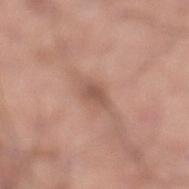Impression: Imaged during a routine full-body skin examination; the lesion was not biopsied and no histopathology is available. Background: The recorded lesion diameter is about 3 mm. Captured under white-light illumination. The lesion is located on the left lower leg. The subject is a male roughly 20 years of age. A 15 mm crop from a total-body photograph taken for skin-cancer surveillance. An algorithmic analysis of the crop reported a lesion area of about 4 mm². The analysis additionally found a border-irregularity rating of about 3/10 and peripheral color asymmetry of about 0.5.